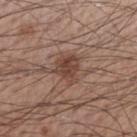The lesion is located on the right forearm. A 15 mm close-up tile from a total-body photography series done for melanoma screening. The patient is a male about 55 years old.